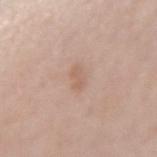Notes:
– follow-up: total-body-photography surveillance lesion; no biopsy
– anatomic site: the right forearm
– illumination: white-light illumination
– size: about 2.5 mm
– subject: female, about 40 years old
– image-analysis metrics: a shape eccentricity near 0.8 and a symmetry-axis asymmetry near 0.2; a lesion-detection confidence of about 100/100
– acquisition: total-body-photography crop, ~15 mm field of view